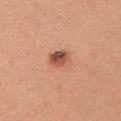Q: Was a biopsy performed?
A: total-body-photography surveillance lesion; no biopsy
Q: Who is the patient?
A: female, aged 38–42
Q: How was the tile lit?
A: white-light
Q: Where on the body is the lesion?
A: the right upper arm
Q: What is the imaging modality?
A: total-body-photography crop, ~15 mm field of view